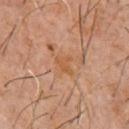Recorded during total-body skin imaging; not selected for excision or biopsy. A 15 mm close-up extracted from a 3D total-body photography capture. The tile uses cross-polarized illumination. The lesion is on the chest. About 3 mm across. The lesion-visualizer software estimated a footprint of about 3.5 mm² and two-axis asymmetry of about 0.35. The software also gave an automated nevus-likeness rating near 0 out of 100 and a detector confidence of about 75 out of 100 that the crop contains a lesion. A male subject aged around 60.